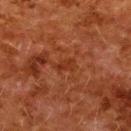Impression: Imaged during a routine full-body skin examination; the lesion was not biopsied and no histopathology is available. Image and clinical context: Automated tile analysis of the lesion measured a lesion area of about 3 mm², an eccentricity of roughly 0.85, and a shape-asymmetry score of about 0.35 (0 = symmetric). A close-up tile cropped from a whole-body skin photograph, about 15 mm across. The lesion's longest dimension is about 2.5 mm. The lesion is located on the upper back. The tile uses cross-polarized illumination. The patient is a female aged around 50.